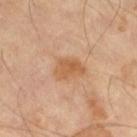A lesion tile, about 15 mm wide, cut from a 3D total-body photograph.
The subject is a male roughly 65 years of age.
The lesion's longest dimension is about 3.5 mm.
This is a cross-polarized tile.
The lesion-visualizer software estimated a nevus-likeness score of about 45/100 and a detector confidence of about 100 out of 100 that the crop contains a lesion.
On the right thigh.